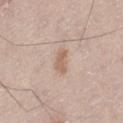workup: no biopsy performed (imaged during a skin exam)
image-analysis metrics: a footprint of about 4 mm², an outline eccentricity of about 0.85 (0 = round, 1 = elongated), and a symmetry-axis asymmetry near 0.35; a lesion color around L≈61 a*≈16 b*≈28 in CIELAB and about 9 CIELAB-L* units darker than the surrounding skin; border irregularity of about 3 on a 0–10 scale and peripheral color asymmetry of about 0.5
patient: male, aged 63 to 67
lighting: white-light
location: the abdomen
acquisition: ~15 mm tile from a whole-body skin photo
size: ≈3 mm